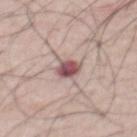Recorded during total-body skin imaging; not selected for excision or biopsy.
The subject is a male roughly 55 years of age.
The lesion is on the mid back.
A close-up tile cropped from a whole-body skin photograph, about 15 mm across.
This is a white-light tile.
Approximately 2.5 mm at its widest.